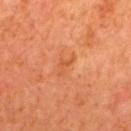Impression: Captured during whole-body skin photography for melanoma surveillance; the lesion was not biopsied. Acquisition and patient details: Automated image analysis of the tile measured a lesion color around L≈55 a*≈30 b*≈43 in CIELAB, a lesion–skin lightness drop of about 6, and a lesion-to-skin contrast of about 5 (normalized; higher = more distinct). It also reported a classifier nevus-likeness of about 0/100. Approximately 3 mm at its widest. A 15 mm crop from a total-body photograph taken for skin-cancer surveillance. This is a cross-polarized tile. The patient is a male approximately 65 years of age. Located on the back.